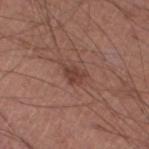Findings:
• tile lighting: white-light illumination
• imaging modality: total-body-photography crop, ~15 mm field of view
• subject: male, aged 43–47
• body site: the right lower leg
• lesion size: ~3 mm (longest diameter)